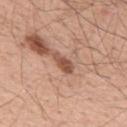Assessment: No biopsy was performed on this lesion — it was imaged during a full skin examination and was not determined to be concerning. Context: The subject is a male aged 53–57. The lesion is on the upper back. A lesion tile, about 15 mm wide, cut from a 3D total-body photograph. The recorded lesion diameter is about 3 mm. Automated tile analysis of the lesion measured a within-lesion color-variation index near 2/10 and a peripheral color-asymmetry measure near 0.5.